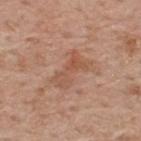| field | value |
|---|---|
| biopsy status | catalogued during a skin exam; not biopsied |
| automated lesion analysis | a footprint of about 7.5 mm², a shape eccentricity near 0.9, and two-axis asymmetry of about 0.35; roughly 7 lightness units darker than nearby skin and a normalized border contrast of about 5.5; border irregularity of about 5.5 on a 0–10 scale and a within-lesion color-variation index near 4/10; an automated nevus-likeness rating near 0 out of 100 and lesion-presence confidence of about 100/100 |
| image source | ~15 mm crop, total-body skin-cancer survey |
| anatomic site | the upper back |
| subject | male, approximately 60 years of age |
| lesion diameter | about 4.5 mm |
| illumination | white-light |